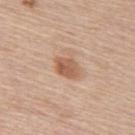The lesion was photographed on a routine skin check and not biopsied; there is no pathology result. The lesion's longest dimension is about 3.5 mm. A female subject roughly 65 years of age. A 15 mm crop from a total-body photograph taken for skin-cancer surveillance. Imaged with white-light lighting. The lesion is located on the upper back.